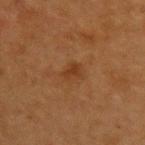No biopsy was performed on this lesion — it was imaged during a full skin examination and was not determined to be concerning. The lesion-visualizer software estimated a lesion color around L≈33 a*≈20 b*≈32 in CIELAB, a lesion–skin lightness drop of about 6, and a lesion-to-skin contrast of about 6 (normalized; higher = more distinct). It also reported a border-irregularity index near 2.5/10, a color-variation rating of about 2/10, and radial color variation of about 0.5. And it measured a classifier nevus-likeness of about 0/100 and lesion-presence confidence of about 100/100. From the upper back. Measured at roughly 2.5 mm in maximum diameter. A 15 mm close-up extracted from a 3D total-body photography capture. A female subject aged 38 to 42.